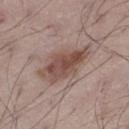| field | value |
|---|---|
| follow-up | imaged on a skin check; not biopsied |
| subject | male, aged 68–72 |
| acquisition | ~15 mm tile from a whole-body skin photo |
| location | the left thigh |